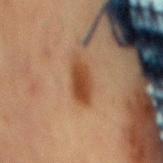Impression: No biopsy was performed on this lesion — it was imaged during a full skin examination and was not determined to be concerning. Acquisition and patient details: Imaged with cross-polarized lighting. A male patient, in their mid- to late 60s. A region of skin cropped from a whole-body photographic capture, roughly 15 mm wide. The lesion-visualizer software estimated a lesion area of about 8 mm² and an outline eccentricity of about 0.9 (0 = round, 1 = elongated). It also reported a border-irregularity index near 3/10, a within-lesion color-variation index near 3/10, and peripheral color asymmetry of about 1. It also reported a classifier nevus-likeness of about 100/100 and a lesion-detection confidence of about 100/100. The lesion is on the abdomen.